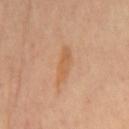Part of a total-body skin-imaging series; this lesion was reviewed on a skin check and was not flagged for biopsy. Cropped from a total-body skin-imaging series; the visible field is about 15 mm. Imaged with cross-polarized lighting. The lesion is located on the mid back. Approximately 4.5 mm at its widest.